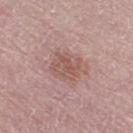biopsy status = imaged on a skin check; not biopsied